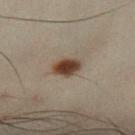Q: Is there a histopathology result?
A: catalogued during a skin exam; not biopsied